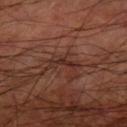Case summary:
- follow-up — no biopsy performed (imaged during a skin exam)
- image — total-body-photography crop, ~15 mm field of view
- location — the right upper arm
- tile lighting — cross-polarized illumination
- automated metrics — a mean CIELAB color near L≈29 a*≈20 b*≈23, a lesion–skin lightness drop of about 7, and a normalized lesion–skin contrast near 7.5; a classifier nevus-likeness of about 0/100
- subject — male, aged approximately 60